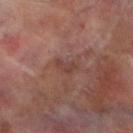Part of a total-body skin-imaging series; this lesion was reviewed on a skin check and was not flagged for biopsy. Imaged with cross-polarized lighting. A male patient, in their 70s. A roughly 15 mm field-of-view crop from a total-body skin photograph. Measured at roughly 2.5 mm in maximum diameter. Located on the left lower leg.